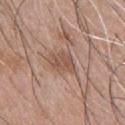Part of a total-body skin-imaging series; this lesion was reviewed on a skin check and was not flagged for biopsy. The lesion is on the front of the torso. Automated image analysis of the tile measured a lesion area of about 7 mm², an eccentricity of roughly 0.7, and a shape-asymmetry score of about 0.25 (0 = symmetric). Captured under white-light illumination. A male patient approximately 45 years of age. A close-up tile cropped from a whole-body skin photograph, about 15 mm across. The lesion's longest dimension is about 3.5 mm.